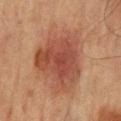| key | value |
|---|---|
| follow-up | imaged on a skin check; not biopsied |
| lesion size | ~8 mm (longest diameter) |
| patient | male, approximately 65 years of age |
| image | ~15 mm tile from a whole-body skin photo |
| body site | the abdomen |